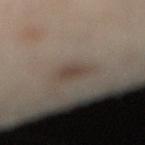Findings:
- follow-up — catalogued during a skin exam; not biopsied
- subject — male, aged around 55
- body site — the right lower leg
- automated metrics — internal color variation of about 1.5 on a 0–10 scale and a peripheral color-asymmetry measure near 0.5
- acquisition — total-body-photography crop, ~15 mm field of view
- tile lighting — cross-polarized illumination